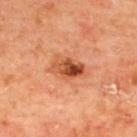Impression:
The lesion was tiled from a total-body skin photograph and was not biopsied.
Context:
A male subject roughly 65 years of age. A close-up tile cropped from a whole-body skin photograph, about 15 mm across. The lesion is located on the back. Captured under cross-polarized illumination. Longest diameter approximately 4 mm.